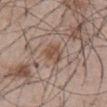| field | value |
|---|---|
| follow-up | imaged on a skin check; not biopsied |
| imaging modality | total-body-photography crop, ~15 mm field of view |
| automated lesion analysis | a lesion–skin lightness drop of about 9; a border-irregularity index near 2.5/10, a within-lesion color-variation index near 3/10, and a peripheral color-asymmetry measure near 1; an automated nevus-likeness rating near 55 out of 100 and a lesion-detection confidence of about 90/100 |
| location | the chest |
| patient | male, approximately 55 years of age |
| lesion size | ~3.5 mm (longest diameter) |
| illumination | white-light |